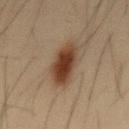diameter — ~6.5 mm (longest diameter) | patient — male, about 35 years old | imaging modality — ~15 mm tile from a whole-body skin photo | anatomic site — the back | lighting — cross-polarized illumination.